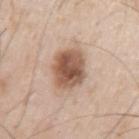Imaged during a routine full-body skin examination; the lesion was not biopsied and no histopathology is available. A roughly 15 mm field-of-view crop from a total-body skin photograph. The subject is a male aged 53–57. Automated tile analysis of the lesion measured a footprint of about 14 mm² and an outline eccentricity of about 0.7 (0 = round, 1 = elongated). The software also gave an automated nevus-likeness rating near 95 out of 100 and a detector confidence of about 100 out of 100 that the crop contains a lesion. The lesion's longest dimension is about 5.5 mm. This is a white-light tile.